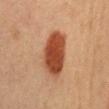Notes:
* follow-up: total-body-photography surveillance lesion; no biopsy
* lesion diameter: ~6 mm (longest diameter)
* tile lighting: cross-polarized
* automated lesion analysis: about 15 CIELAB-L* units darker than the surrounding skin and a normalized border contrast of about 11.5; border irregularity of about 2 on a 0–10 scale, a within-lesion color-variation index near 3.5/10, and peripheral color asymmetry of about 1
* image: 15 mm crop, total-body photography
* subject: female, about 40 years old
* location: the abdomen